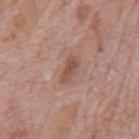Imaged during a routine full-body skin examination; the lesion was not biopsied and no histopathology is available. Located on the mid back. A male subject, about 75 years old. A 15 mm close-up tile from a total-body photography series done for melanoma screening.